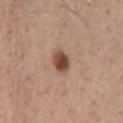follow-up=total-body-photography surveillance lesion; no biopsy
size=about 3 mm
subject=male, roughly 55 years of age
body site=the lower back
image source=total-body-photography crop, ~15 mm field of view